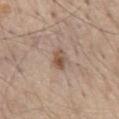Recorded during total-body skin imaging; not selected for excision or biopsy.
Located on the mid back.
The tile uses white-light illumination.
A male subject in their mid-60s.
The recorded lesion diameter is about 2.5 mm.
Cropped from a total-body skin-imaging series; the visible field is about 15 mm.